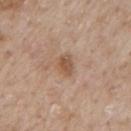This lesion was catalogued during total-body skin photography and was not selected for biopsy. Located on the back. The subject is a male aged 63 to 67. The total-body-photography lesion software estimated a lesion color around L≈53 a*≈19 b*≈30 in CIELAB and about 9 CIELAB-L* units darker than the surrounding skin. It also reported a nevus-likeness score of about 10/100. A 15 mm crop from a total-body photograph taken for skin-cancer surveillance.